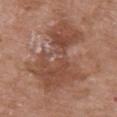The lesion-visualizer software estimated a footprint of about 41 mm², an eccentricity of roughly 0.85, and a shape-asymmetry score of about 0.45 (0 = symmetric). The software also gave an automated nevus-likeness rating near 0 out of 100 and lesion-presence confidence of about 100/100. Cropped from a whole-body photographic skin survey; the tile spans about 15 mm. A female subject, about 70 years old. Longest diameter approximately 10.5 mm. Located on the right upper arm. This is a white-light tile.